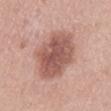workup: imaged on a skin check; not biopsied | illumination: white-light illumination | image source: 15 mm crop, total-body photography | location: the back | TBP lesion metrics: an area of roughly 25 mm² and a shape-asymmetry score of about 0.1 (0 = symmetric); a lesion color around L≈55 a*≈23 b*≈25 in CIELAB, a lesion–skin lightness drop of about 14, and a normalized lesion–skin contrast near 9; a border-irregularity index near 2/10 and internal color variation of about 4 on a 0–10 scale; a detector confidence of about 100 out of 100 that the crop contains a lesion | subject: male, in their mid-50s.